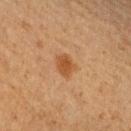Q: Was this lesion biopsied?
A: no biopsy performed (imaged during a skin exam)
Q: What is the imaging modality?
A: ~15 mm tile from a whole-body skin photo
Q: Lesion location?
A: the left upper arm
Q: Patient demographics?
A: male, aged approximately 60